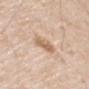Imaged during a routine full-body skin examination; the lesion was not biopsied and no histopathology is available. The total-body-photography lesion software estimated a lesion area of about 6 mm², an outline eccentricity of about 0.7 (0 = round, 1 = elongated), and two-axis asymmetry of about 0.2. The software also gave an average lesion color of about L≈65 a*≈17 b*≈32 (CIELAB), a lesion–skin lightness drop of about 10, and a lesion-to-skin contrast of about 6.5 (normalized; higher = more distinct). The software also gave a classifier nevus-likeness of about 25/100. The recorded lesion diameter is about 3 mm. A roughly 15 mm field-of-view crop from a total-body skin photograph. A male subject, aged 63–67. From the left upper arm.